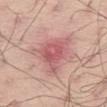| feature | finding |
|---|---|
| follow-up | catalogued during a skin exam; not biopsied |
| image | total-body-photography crop, ~15 mm field of view |
| anatomic site | the left thigh |
| subject | male, in their mid-40s |
| lesion size | about 6 mm |
| illumination | white-light illumination |